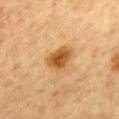Assessment: This lesion was catalogued during total-body skin photography and was not selected for biopsy. Context: The lesion is on the mid back. Automated image analysis of the tile measured a footprint of about 8 mm² and a shape eccentricity near 0.7. The software also gave about 12 CIELAB-L* units darker than the surrounding skin and a normalized border contrast of about 9. It also reported a nevus-likeness score of about 100/100 and a lesion-detection confidence of about 100/100. Approximately 4 mm at its widest. This is a cross-polarized tile. The subject is a female aged around 50. Cropped from a total-body skin-imaging series; the visible field is about 15 mm.